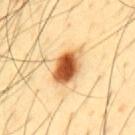{
  "biopsy_status": "not biopsied; imaged during a skin examination",
  "image": {
    "source": "total-body photography crop",
    "field_of_view_mm": 15
  },
  "site": "mid back",
  "lesion_size": {
    "long_diameter_mm_approx": 4.5
  },
  "lighting": "cross-polarized",
  "patient": {
    "sex": "male",
    "age_approx": 45
  }
}